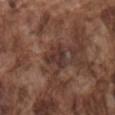Q: What is the anatomic site?
A: the mid back
Q: What is the lesion's diameter?
A: ~3 mm (longest diameter)
Q: What are the patient's age and sex?
A: male, aged around 75
Q: What kind of image is this?
A: ~15 mm crop, total-body skin-cancer survey
Q: Automated lesion metrics?
A: a footprint of about 6.5 mm² and an outline eccentricity of about 0.2 (0 = round, 1 = elongated); a within-lesion color-variation index near 3/10; a lesion-detection confidence of about 85/100
Q: How was the tile lit?
A: white-light illumination